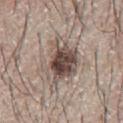biopsy status = imaged on a skin check; not biopsied.